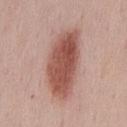- lesion size — ~8.5 mm (longest diameter)
- patient — male, aged approximately 50
- acquisition — ~15 mm tile from a whole-body skin photo
- illumination — white-light
- location — the mid back